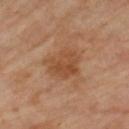<lesion>
<biopsy_status>not biopsied; imaged during a skin examination</biopsy_status>
<patient>
  <sex>female</sex>
  <age_approx>50</age_approx>
</patient>
<image>
  <source>total-body photography crop</source>
  <field_of_view_mm>15</field_of_view_mm>
</image>
<site>right lower leg</site>
</lesion>